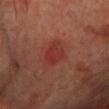This lesion was catalogued during total-body skin photography and was not selected for biopsy. An algorithmic analysis of the crop reported a shape eccentricity near 0.7 and a shape-asymmetry score of about 0.3 (0 = symmetric). And it measured border irregularity of about 2.5 on a 0–10 scale, a within-lesion color-variation index near 2.5/10, and radial color variation of about 1. The software also gave a nevus-likeness score of about 0/100 and lesion-presence confidence of about 100/100. A 15 mm close-up tile from a total-body photography series done for melanoma screening. The lesion is located on the chest. Captured under cross-polarized illumination. A male patient, approximately 70 years of age. The lesion's longest dimension is about 3.5 mm.